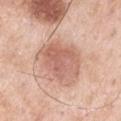Clinical summary:
Cropped from a whole-body photographic skin survey; the tile spans about 15 mm. A male subject, aged 53 to 57. From the right upper arm.